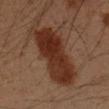Assessment: Imaged during a routine full-body skin examination; the lesion was not biopsied and no histopathology is available. Background: From the left upper arm. A male patient in their 50s. A region of skin cropped from a whole-body photographic capture, roughly 15 mm wide.